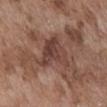diameter = ≈4.5 mm
lighting = white-light illumination
subject = male, aged around 75
image-analysis metrics = a lesion area of about 12 mm², an eccentricity of roughly 0.5, and two-axis asymmetry of about 0.5
body site = the front of the torso
imaging modality = ~15 mm crop, total-body skin-cancer survey